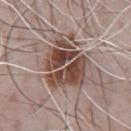<lesion>
<biopsy_status>not biopsied; imaged during a skin examination</biopsy_status>
<site>chest</site>
<image>
  <source>total-body photography crop</source>
  <field_of_view_mm>15</field_of_view_mm>
</image>
<lesion_size>
  <long_diameter_mm_approx>6.5</long_diameter_mm_approx>
</lesion_size>
<patient>
  <sex>male</sex>
  <age_approx>70</age_approx>
</patient>
<automated_metrics>
  <cielab_L>46</cielab_L>
  <cielab_a>18</cielab_a>
  <cielab_b>23</cielab_b>
  <vs_skin_darker_L>14.0</vs_skin_darker_L>
  <vs_skin_contrast_norm>10.5</vs_skin_contrast_norm>
  <nevus_likeness_0_100>95</nevus_likeness_0_100>
  <lesion_detection_confidence_0_100>100</lesion_detection_confidence_0_100>
</automated_metrics>
<lighting>white-light</lighting>
</lesion>